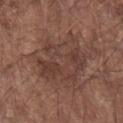{"biopsy_status": "not biopsied; imaged during a skin examination"}